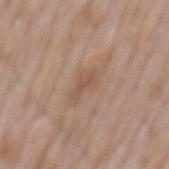<case>
  <biopsy_status>not biopsied; imaged during a skin examination</biopsy_status>
  <site>mid back</site>
  <image>
    <source>total-body photography crop</source>
    <field_of_view_mm>15</field_of_view_mm>
  </image>
  <patient>
    <sex>male</sex>
    <age_approx>60</age_approx>
  </patient>
</case>